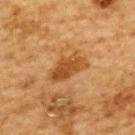- workup · catalogued during a skin exam; not biopsied
- subject · male, aged 83 or older
- site · the upper back
- image source · 15 mm crop, total-body photography